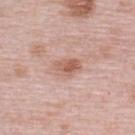body site — the upper back
automated lesion analysis — an area of roughly 5 mm², a shape eccentricity near 0.75, and two-axis asymmetry of about 0.3; an average lesion color of about L≈59 a*≈22 b*≈28 (CIELAB), roughly 10 lightness units darker than nearby skin, and a normalized lesion–skin contrast near 7; a nevus-likeness score of about 10/100 and a lesion-detection confidence of about 100/100
image source — 15 mm crop, total-body photography
subject — female, in their mid-60s